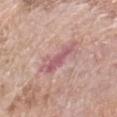This lesion was catalogued during total-body skin photography and was not selected for biopsy.
A female patient, in their mid- to late 70s.
A 15 mm close-up tile from a total-body photography series done for melanoma screening.
An algorithmic analysis of the crop reported border irregularity of about 5 on a 0–10 scale, a within-lesion color-variation index near 3.5/10, and a peripheral color-asymmetry measure near 1.
The lesion is on the left forearm.